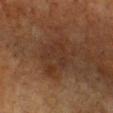Part of a total-body skin-imaging series; this lesion was reviewed on a skin check and was not flagged for biopsy. A roughly 15 mm field-of-view crop from a total-body skin photograph. On the chest. A female patient aged 53–57.